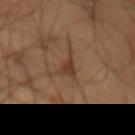{"biopsy_status": "not biopsied; imaged during a skin examination", "lighting": "cross-polarized", "image": {"source": "total-body photography crop", "field_of_view_mm": 15}, "lesion_size": {"long_diameter_mm_approx": 4.0}, "patient": {"sex": "male", "age_approx": 60}, "site": "front of the torso"}